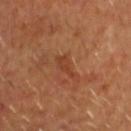follow-up: catalogued during a skin exam; not biopsied.